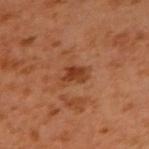Q: Is there a histopathology result?
A: no biopsy performed (imaged during a skin exam)
Q: Lesion size?
A: ≈3 mm
Q: What kind of image is this?
A: total-body-photography crop, ~15 mm field of view
Q: Automated lesion metrics?
A: a lesion color around L≈38 a*≈24 b*≈33 in CIELAB, about 9 CIELAB-L* units darker than the surrounding skin, and a normalized border contrast of about 7.5
Q: Where on the body is the lesion?
A: the right upper arm
Q: What are the patient's age and sex?
A: male, in their 60s
Q: How was the tile lit?
A: cross-polarized